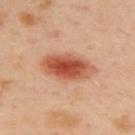Captured during whole-body skin photography for melanoma surveillance; the lesion was not biopsied. The lesion is located on the back. The patient is a female aged 38 to 42. A 15 mm crop from a total-body photograph taken for skin-cancer surveillance. About 6.5 mm across. The lesion-visualizer software estimated a border-irregularity index near 2/10 and internal color variation of about 7 on a 0–10 scale. The software also gave a nevus-likeness score of about 100/100 and a lesion-detection confidence of about 100/100. Imaged with cross-polarized lighting.